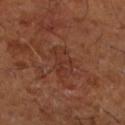biopsy status: imaged on a skin check; not biopsied | body site: the left lower leg | imaging modality: ~15 mm crop, total-body skin-cancer survey | size: about 4 mm | lighting: cross-polarized | automated metrics: an outline eccentricity of about 0.85 (0 = round, 1 = elongated) and a symmetry-axis asymmetry near 0.35; a lesion color around L≈34 a*≈23 b*≈28 in CIELAB, roughly 5 lightness units darker than nearby skin, and a lesion-to-skin contrast of about 5 (normalized; higher = more distinct); a within-lesion color-variation index near 2.5/10 and radial color variation of about 1 | subject: male, aged around 65.